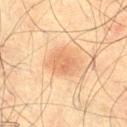A male subject about 50 years old.
This is a cross-polarized tile.
Automated image analysis of the tile measured a lesion color around L≈54 a*≈20 b*≈32 in CIELAB, roughly 7 lightness units darker than nearby skin, and a normalized border contrast of about 5. And it measured a border-irregularity index near 2/10, internal color variation of about 2.5 on a 0–10 scale, and a peripheral color-asymmetry measure near 1.
Cropped from a whole-body photographic skin survey; the tile spans about 15 mm.
The lesion is located on the right thigh.
Approximately 2.5 mm at its widest.